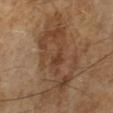| feature | finding |
|---|---|
| notes | catalogued during a skin exam; not biopsied |
| illumination | cross-polarized illumination |
| diameter | ≈11 mm |
| image source | total-body-photography crop, ~15 mm field of view |
| patient | male, approximately 65 years of age |